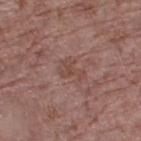Background: A female patient aged 73 to 77. The lesion is located on the right thigh. A lesion tile, about 15 mm wide, cut from a 3D total-body photograph.